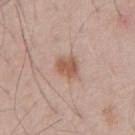This lesion was catalogued during total-body skin photography and was not selected for biopsy.
This is a white-light tile.
A region of skin cropped from a whole-body photographic capture, roughly 15 mm wide.
A male patient roughly 65 years of age.
From the front of the torso.
Longest diameter approximately 2.5 mm.